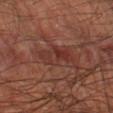The lesion was photographed on a routine skin check and not biopsied; there is no pathology result. The total-body-photography lesion software estimated an average lesion color of about L≈30 a*≈22 b*≈22 (CIELAB) and a lesion-to-skin contrast of about 6 (normalized; higher = more distinct). The analysis additionally found border irregularity of about 4.5 on a 0–10 scale, a color-variation rating of about 6/10, and a peripheral color-asymmetry measure near 2. And it measured an automated nevus-likeness rating near 0 out of 100 and lesion-presence confidence of about 80/100. A male subject, approximately 60 years of age. The lesion is located on the right lower leg. A region of skin cropped from a whole-body photographic capture, roughly 15 mm wide. The recorded lesion diameter is about 5.5 mm. This is a cross-polarized tile.